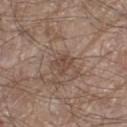<record>
<biopsy_status>not biopsied; imaged during a skin examination</biopsy_status>
</record>